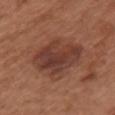<lesion>
  <biopsy_status>not biopsied; imaged during a skin examination</biopsy_status>
  <patient>
    <sex>female</sex>
    <age_approx>65</age_approx>
  </patient>
  <site>chest</site>
  <lesion_size>
    <long_diameter_mm_approx>6.5</long_diameter_mm_approx>
  </lesion_size>
  <image>
    <source>total-body photography crop</source>
    <field_of_view_mm>15</field_of_view_mm>
  </image>
</lesion>